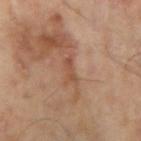<lesion>
<lighting>cross-polarized</lighting>
<site>right upper arm</site>
<lesion_size>
  <long_diameter_mm_approx>3.0</long_diameter_mm_approx>
</lesion_size>
<patient>
  <sex>male</sex>
  <age_approx>65</age_approx>
</patient>
<automated_metrics>
  <area_mm2_approx>3.0</area_mm2_approx>
  <eccentricity>0.9</eccentricity>
  <shape_asymmetry>0.3</shape_asymmetry>
  <cielab_L>47</cielab_L>
  <cielab_a>21</cielab_a>
  <cielab_b>30</cielab_b>
  <vs_skin_darker_L>7.0</vs_skin_darker_L>
  <vs_skin_contrast_norm>6.0</vs_skin_contrast_norm>
  <border_irregularity_0_10>4.5</border_irregularity_0_10>
  <color_variation_0_10>0.0</color_variation_0_10>
  <nevus_likeness_0_100>0</nevus_likeness_0_100>
</automated_metrics>
<image>
  <source>total-body photography crop</source>
  <field_of_view_mm>15</field_of_view_mm>
</image>
</lesion>